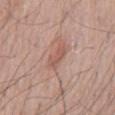Impression:
Part of a total-body skin-imaging series; this lesion was reviewed on a skin check and was not flagged for biopsy.
Clinical summary:
The total-body-photography lesion software estimated a mean CIELAB color near L≈55 a*≈23 b*≈26 and a lesion–skin lightness drop of about 8. It also reported a border-irregularity rating of about 3/10, internal color variation of about 0 on a 0–10 scale, and radial color variation of about 0. This is a white-light tile. The lesion's longest dimension is about 3 mm. A male patient, in their 60s. Located on the abdomen. A close-up tile cropped from a whole-body skin photograph, about 15 mm across.